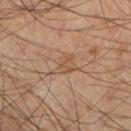| key | value |
|---|---|
| location | the left lower leg |
| diameter | ~3 mm (longest diameter) |
| patient | male, in their 40s |
| imaging modality | total-body-photography crop, ~15 mm field of view |
| automated metrics | a lesion color around L≈53 a*≈18 b*≈32 in CIELAB, roughly 7 lightness units darker than nearby skin, and a normalized lesion–skin contrast near 5.5; a border-irregularity index near 5/10, a within-lesion color-variation index near 2/10, and a peripheral color-asymmetry measure near 1; a nevus-likeness score of about 0/100 and lesion-presence confidence of about 95/100 |